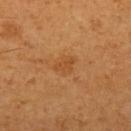Recorded during total-body skin imaging; not selected for excision or biopsy.